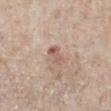Notes:
• biopsy status · total-body-photography surveillance lesion; no biopsy
• tile lighting · white-light
• lesion size · ≈3 mm
• site · the leg
• patient · female, aged approximately 55
• acquisition · ~15 mm tile from a whole-body skin photo